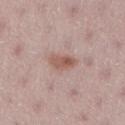lesion diameter: about 3.5 mm | patient: female, aged 23–27 | anatomic site: the right lower leg | image source: total-body-photography crop, ~15 mm field of view.